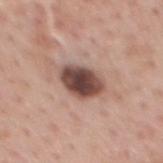biopsy_status: not biopsied; imaged during a skin examination
lighting: white-light
automated_metrics:
  area_mm2_approx: 11.0
  eccentricity: 0.7
  shape_asymmetry: 0.2
  nevus_likeness_0_100: 80
  lesion_detection_confidence_0_100: 100
lesion_size:
  long_diameter_mm_approx: 4.5
image:
  source: total-body photography crop
  field_of_view_mm: 15
patient:
  sex: male
  age_approx: 60
site: mid back